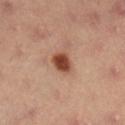No biopsy was performed on this lesion — it was imaged during a full skin examination and was not determined to be concerning.
On the leg.
A female patient, in their mid- to late 30s.
A roughly 15 mm field-of-view crop from a total-body skin photograph.
The recorded lesion diameter is about 2.5 mm.
This is a cross-polarized tile.
Automated tile analysis of the lesion measured a lesion area of about 5 mm², an outline eccentricity of about 0.45 (0 = round, 1 = elongated), and a shape-asymmetry score of about 0.2 (0 = symmetric). And it measured border irregularity of about 2 on a 0–10 scale, a color-variation rating of about 3.5/10, and radial color variation of about 1.